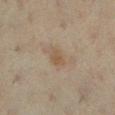No biopsy was performed on this lesion — it was imaged during a full skin examination and was not determined to be concerning.
Captured under cross-polarized illumination.
The recorded lesion diameter is about 2.5 mm.
On the left lower leg.
A female patient, in their mid- to late 60s.
A 15 mm close-up tile from a total-body photography series done for melanoma screening.
An algorithmic analysis of the crop reported an eccentricity of roughly 0.75 and two-axis asymmetry of about 0.35. It also reported lesion-presence confidence of about 100/100.